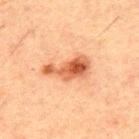<record>
<biopsy_status>not biopsied; imaged during a skin examination</biopsy_status>
<site>back</site>
<lesion_size>
  <long_diameter_mm_approx>5.5</long_diameter_mm_approx>
</lesion_size>
<image>
  <source>total-body photography crop</source>
  <field_of_view_mm>15</field_of_view_mm>
</image>
<lighting>cross-polarized</lighting>
<patient>
  <sex>male</sex>
  <age_approx>50</age_approx>
</patient>
</record>